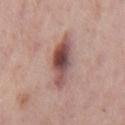Imaged during a routine full-body skin examination; the lesion was not biopsied and no histopathology is available. A lesion tile, about 15 mm wide, cut from a 3D total-body photograph. The tile uses white-light illumination. A male subject roughly 75 years of age. On the mid back. About 5.5 mm across.